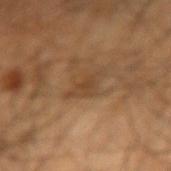Clinical impression: The lesion was tiled from a total-body skin photograph and was not biopsied. Acquisition and patient details: On the right upper arm. The subject is a male aged approximately 45. Captured under cross-polarized illumination. A 15 mm crop from a total-body photograph taken for skin-cancer surveillance. The lesion-visualizer software estimated an area of roughly 6 mm², a shape eccentricity near 0.85, and two-axis asymmetry of about 0.3. The analysis additionally found an average lesion color of about L≈41 a*≈17 b*≈31 (CIELAB), roughly 6 lightness units darker than nearby skin, and a lesion-to-skin contrast of about 5.5 (normalized; higher = more distinct). The analysis additionally found lesion-presence confidence of about 95/100. The recorded lesion diameter is about 4 mm.